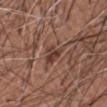{"biopsy_status": "not biopsied; imaged during a skin examination", "lesion_size": {"long_diameter_mm_approx": 3.5}, "image": {"source": "total-body photography crop", "field_of_view_mm": 15}, "site": "left forearm", "patient": {"sex": "male", "age_approx": 60}}